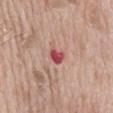{"biopsy_status": "not biopsied; imaged during a skin examination", "lesion_size": {"long_diameter_mm_approx": 2.5}, "image": {"source": "total-body photography crop", "field_of_view_mm": 15}, "automated_metrics": {"area_mm2_approx": 3.0, "eccentricity": 0.75, "shape_asymmetry": 0.3}, "patient": {"sex": "male", "age_approx": 65}, "site": "mid back"}